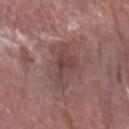Notes:
• biopsy status · no biopsy performed (imaged during a skin exam)
• body site · the left forearm
• lesion size · ≈5 mm
• illumination · white-light illumination
• image · ~15 mm tile from a whole-body skin photo
• subject · male, about 80 years old